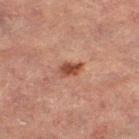Context: The total-body-photography lesion software estimated a mean CIELAB color near L≈39 a*≈22 b*≈27, roughly 11 lightness units darker than nearby skin, and a normalized lesion–skin contrast near 9.5. And it measured an automated nevus-likeness rating near 95 out of 100. Imaged with cross-polarized lighting. On the left thigh. A female subject in their 70s. Longest diameter approximately 2.5 mm. This image is a 15 mm lesion crop taken from a total-body photograph.